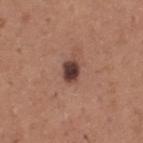biopsy_status: not biopsied; imaged during a skin examination
patient:
  sex: male
  age_approx: 65
image:
  source: total-body photography crop
  field_of_view_mm: 15
site: upper back